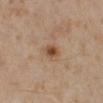Imaged during a routine full-body skin examination; the lesion was not biopsied and no histopathology is available. The subject is a female roughly 40 years of age. The lesion's longest dimension is about 2.5 mm. Captured under cross-polarized illumination. From the leg. This image is a 15 mm lesion crop taken from a total-body photograph. An algorithmic analysis of the crop reported an outline eccentricity of about 0.65 (0 = round, 1 = elongated) and two-axis asymmetry of about 0.15.